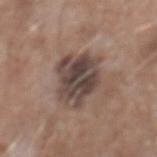Case summary:
- workup · imaged on a skin check; not biopsied
- lesion diameter · about 6 mm
- acquisition · 15 mm crop, total-body photography
- TBP lesion metrics · an area of roughly 20 mm² and a shape-asymmetry score of about 0.25 (0 = symmetric)
- patient · male, roughly 60 years of age
- site · the mid back
- lighting · white-light